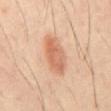The lesion was photographed on a routine skin check and not biopsied; there is no pathology result. A male patient aged 38–42. Located on the abdomen. A 15 mm close-up extracted from a 3D total-body photography capture. About 5.5 mm across.